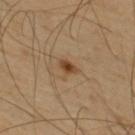The lesion was tiled from a total-body skin photograph and was not biopsied.
Automated image analysis of the tile measured a lesion area of about 3 mm² and an outline eccentricity of about 0.85 (0 = round, 1 = elongated). It also reported an average lesion color of about L≈43 a*≈19 b*≈34 (CIELAB), about 11 CIELAB-L* units darker than the surrounding skin, and a lesion-to-skin contrast of about 9.5 (normalized; higher = more distinct). It also reported a detector confidence of about 100 out of 100 that the crop contains a lesion.
Located on the upper back.
The tile uses cross-polarized illumination.
Cropped from a total-body skin-imaging series; the visible field is about 15 mm.
A male patient, roughly 65 years of age.